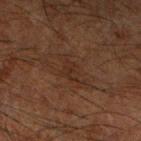<tbp_lesion>
  <biopsy_status>not biopsied; imaged during a skin examination</biopsy_status>
</tbp_lesion>